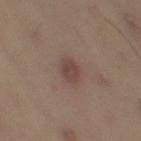Imaged during a routine full-body skin examination; the lesion was not biopsied and no histopathology is available. A roughly 15 mm field-of-view crop from a total-body skin photograph. On the left thigh. A female patient, aged approximately 45. Imaged with cross-polarized lighting. The lesion's longest dimension is about 2.5 mm.